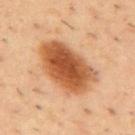Recorded during total-body skin imaging; not selected for excision or biopsy. The subject is a male in their mid-50s. An algorithmic analysis of the crop reported a mean CIELAB color near L≈54 a*≈25 b*≈39, a lesion–skin lightness drop of about 16, and a normalized lesion–skin contrast near 11. The software also gave a border-irregularity index near 2/10, a color-variation rating of about 6.5/10, and a peripheral color-asymmetry measure near 2. Located on the upper back. A roughly 15 mm field-of-view crop from a total-body skin photograph.